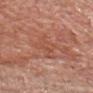Captured during whole-body skin photography for melanoma surveillance; the lesion was not biopsied. From the head or neck. The lesion's longest dimension is about 3.5 mm. A 15 mm crop from a total-body photograph taken for skin-cancer surveillance. The subject is a male approximately 80 years of age. An algorithmic analysis of the crop reported a border-irregularity rating of about 5/10, a color-variation rating of about 2.5/10, and a peripheral color-asymmetry measure near 1. It also reported lesion-presence confidence of about 95/100. Imaged with white-light lighting.